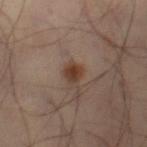Q: Was a biopsy performed?
A: no biopsy performed (imaged during a skin exam)
Q: What are the patient's age and sex?
A: male, in their 50s
Q: How was the tile lit?
A: cross-polarized illumination
Q: What kind of image is this?
A: 15 mm crop, total-body photography
Q: Lesion location?
A: the left leg
Q: What did automated image analysis measure?
A: an area of roughly 4 mm² and two-axis asymmetry of about 0.25; an average lesion color of about L≈38 a*≈17 b*≈26 (CIELAB) and a normalized border contrast of about 9
Q: What is the lesion's diameter?
A: ≈2.5 mm